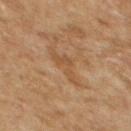biopsy status = catalogued during a skin exam; not biopsied
subject = female, roughly 60 years of age
imaging modality = total-body-photography crop, ~15 mm field of view
lesion size = about 4.5 mm
anatomic site = the upper back